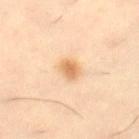Q: Was this lesion biopsied?
A: total-body-photography surveillance lesion; no biopsy
Q: Who is the patient?
A: female, aged approximately 45
Q: What is the imaging modality?
A: total-body-photography crop, ~15 mm field of view
Q: What is the anatomic site?
A: the right thigh